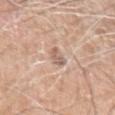Impression:
Part of a total-body skin-imaging series; this lesion was reviewed on a skin check and was not flagged for biopsy.
Context:
The total-body-photography lesion software estimated a footprint of about 3.5 mm², an outline eccentricity of about 0.75 (0 = round, 1 = elongated), and two-axis asymmetry of about 0.3. The software also gave a mean CIELAB color near L≈61 a*≈17 b*≈26, a lesion–skin lightness drop of about 9, and a normalized lesion–skin contrast near 6. The analysis additionally found a border-irregularity index near 3/10, internal color variation of about 2 on a 0–10 scale, and a peripheral color-asymmetry measure near 0.5. The subject is a male roughly 60 years of age. On the left upper arm. The tile uses white-light illumination. Longest diameter approximately 2.5 mm. A 15 mm close-up tile from a total-body photography series done for melanoma screening.